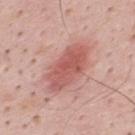Findings:
- follow-up · catalogued during a skin exam; not biopsied
- patient · male, aged approximately 35
- site · the mid back
- image source · ~15 mm crop, total-body skin-cancer survey